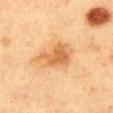Captured during whole-body skin photography for melanoma surveillance; the lesion was not biopsied.
On the left thigh.
This is a cross-polarized tile.
Measured at roughly 5 mm in maximum diameter.
The patient is a female approximately 40 years of age.
A lesion tile, about 15 mm wide, cut from a 3D total-body photograph.
The lesion-visualizer software estimated a lesion area of about 12 mm², an eccentricity of roughly 0.8, and a symmetry-axis asymmetry near 0.3. The software also gave a mean CIELAB color near L≈56 a*≈20 b*≈39, roughly 10 lightness units darker than nearby skin, and a normalized lesion–skin contrast near 7.5. It also reported border irregularity of about 3.5 on a 0–10 scale, a within-lesion color-variation index near 4.5/10, and peripheral color asymmetry of about 1.5.